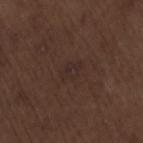follow-up=no biopsy performed (imaged during a skin exam)
lesion size=≈3 mm
acquisition=15 mm crop, total-body photography
tile lighting=white-light
subject=male, in their 70s
automated lesion analysis=an eccentricity of roughly 0.75; a border-irregularity index near 3/10, a color-variation rating of about 2/10, and a peripheral color-asymmetry measure near 0.5
site=the lower back